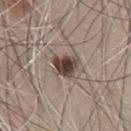| field | value |
|---|---|
| diameter | ~3 mm (longest diameter) |
| illumination | white-light illumination |
| patient | male, aged 63 to 67 |
| location | the abdomen |
| imaging modality | ~15 mm crop, total-body skin-cancer survey |